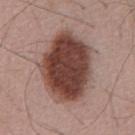Assessment: Captured during whole-body skin photography for melanoma surveillance; the lesion was not biopsied. Acquisition and patient details: The patient is a male aged approximately 55. Approximately 8.5 mm at its widest. This image is a 15 mm lesion crop taken from a total-body photograph.